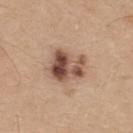This lesion was catalogued during total-body skin photography and was not selected for biopsy.
Captured under white-light illumination.
The subject is a male aged 33 to 37.
A roughly 15 mm field-of-view crop from a total-body skin photograph.
The total-body-photography lesion software estimated a lesion area of about 12 mm², a shape eccentricity near 0.65, and a shape-asymmetry score of about 0.35 (0 = symmetric). It also reported border irregularity of about 4 on a 0–10 scale, a within-lesion color-variation index near 9.5/10, and a peripheral color-asymmetry measure near 5.
The recorded lesion diameter is about 4.5 mm.
From the upper back.